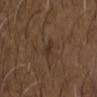  image:
    source: total-body photography crop
    field_of_view_mm: 15
  lesion_size:
    long_diameter_mm_approx: 2.5
  automated_metrics:
    cielab_L: 31
    cielab_a: 14
    cielab_b: 22
    vs_skin_darker_L: 6.0
    vs_skin_contrast_norm: 7.5
    border_irregularity_0_10: 3.0
    color_variation_0_10: 1.5
    peripheral_color_asymmetry: 0.5
  patient:
    sex: male
    age_approx: 50
  lighting: white-light
  site: chest